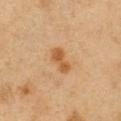Impression: No biopsy was performed on this lesion — it was imaged during a full skin examination and was not determined to be concerning. Background: A male subject, aged approximately 50. The lesion is on the right upper arm. A 15 mm close-up extracted from a 3D total-body photography capture.